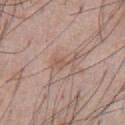Q: Is there a histopathology result?
A: total-body-photography surveillance lesion; no biopsy